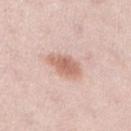The lesion's longest dimension is about 4 mm. A female subject approximately 30 years of age. Located on the right lower leg. Automated image analysis of the tile measured an area of roughly 7.5 mm², an outline eccentricity of about 0.8 (0 = round, 1 = elongated), and a symmetry-axis asymmetry near 0.2. The analysis additionally found a lesion color around L≈64 a*≈22 b*≈28 in CIELAB and a normalized lesion–skin contrast near 7.5. The software also gave a border-irregularity rating of about 2.5/10. The analysis additionally found a nevus-likeness score of about 90/100 and a detector confidence of about 100 out of 100 that the crop contains a lesion. A 15 mm crop from a total-body photograph taken for skin-cancer surveillance. Captured under white-light illumination.